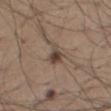A male subject, aged 63–67. The lesion is on the right thigh. A 15 mm close-up extracted from a 3D total-body photography capture.